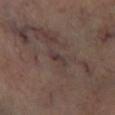Recorded during total-body skin imaging; not selected for excision or biopsy.
Cropped from a total-body skin-imaging series; the visible field is about 15 mm.
The tile uses cross-polarized illumination.
Approximately 2.5 mm at its widest.
From the right lower leg.
The total-body-photography lesion software estimated a footprint of about 2.5 mm² and a symmetry-axis asymmetry near 0.4.